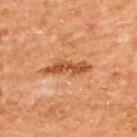{
  "biopsy_status": "not biopsied; imaged during a skin examination",
  "automated_metrics": {
    "cielab_L": 48,
    "cielab_a": 25,
    "cielab_b": 38,
    "vs_skin_darker_L": 11.0,
    "vs_skin_contrast_norm": 8.5
  },
  "lighting": "cross-polarized",
  "image": {
    "source": "total-body photography crop",
    "field_of_view_mm": 15
  },
  "patient": {
    "sex": "male",
    "age_approx": 65
  },
  "lesion_size": {
    "long_diameter_mm_approx": 5.5
  },
  "site": "upper back"
}